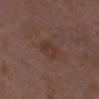Q: Was this lesion biopsied?
A: no biopsy performed (imaged during a skin exam)
Q: How was this image acquired?
A: 15 mm crop, total-body photography
Q: Lesion location?
A: the chest
Q: Patient demographics?
A: female, about 30 years old